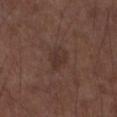Case summary:
* notes · imaged on a skin check; not biopsied
* lighting · white-light
* acquisition · ~15 mm crop, total-body skin-cancer survey
* location · the right forearm
* patient · male, in their mid-70s
* lesion diameter · about 3 mm